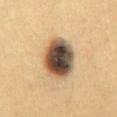No biopsy was performed on this lesion — it was imaged during a full skin examination and was not determined to be concerning. A female subject roughly 30 years of age. A 15 mm close-up tile from a total-body photography series done for melanoma screening. Located on the mid back. Imaged with cross-polarized lighting.